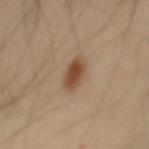<case>
  <biopsy_status>not biopsied; imaged during a skin examination</biopsy_status>
  <image>
    <source>total-body photography crop</source>
    <field_of_view_mm>15</field_of_view_mm>
  </image>
  <site>mid back</site>
  <patient>
    <sex>male</sex>
    <age_approx>45</age_approx>
  </patient>
  <automated_metrics>
    <area_mm2_approx>7.0</area_mm2_approx>
    <eccentricity>0.8</eccentricity>
    <cielab_L>47</cielab_L>
    <cielab_a>18</cielab_a>
    <cielab_b>30</cielab_b>
    <vs_skin_darker_L>11.0</vs_skin_darker_L>
    <vs_skin_contrast_norm>9.0</vs_skin_contrast_norm>
    <nevus_likeness_0_100>100</nevus_likeness_0_100>
  </automated_metrics>
  <lighting>cross-polarized</lighting>
  <lesion_size>
    <long_diameter_mm_approx>3.5</long_diameter_mm_approx>
  </lesion_size>
</case>